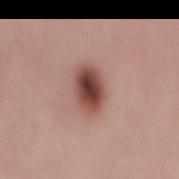– notes — imaged on a skin check; not biopsied
– illumination — white-light
– acquisition — total-body-photography crop, ~15 mm field of view
– patient — male, aged approximately 30
– body site — the mid back